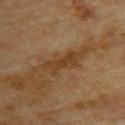Q: Was a biopsy performed?
A: imaged on a skin check; not biopsied
Q: Where on the body is the lesion?
A: the upper back
Q: Patient demographics?
A: female, aged 78–82
Q: What kind of image is this?
A: ~15 mm crop, total-body skin-cancer survey
Q: What did automated image analysis measure?
A: a lesion color around L≈33 a*≈17 b*≈30 in CIELAB and roughly 7 lightness units darker than nearby skin
Q: How large is the lesion?
A: ≈5 mm